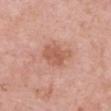| field | value |
|---|---|
| follow-up | no biopsy performed (imaged during a skin exam) |
| anatomic site | the chest |
| subject | female, in their mid- to late 60s |
| acquisition | 15 mm crop, total-body photography |